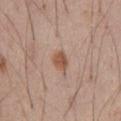The lesion was tiled from a total-body skin photograph and was not biopsied. The tile uses white-light illumination. Located on the abdomen. Approximately 2.5 mm at its widest. The total-body-photography lesion software estimated a mean CIELAB color near L≈53 a*≈21 b*≈29, about 11 CIELAB-L* units darker than the surrounding skin, and a lesion-to-skin contrast of about 8 (normalized; higher = more distinct). The analysis additionally found a border-irregularity rating of about 1.5/10, internal color variation of about 2.5 on a 0–10 scale, and peripheral color asymmetry of about 1. A male patient, aged 58–62. This image is a 15 mm lesion crop taken from a total-body photograph.